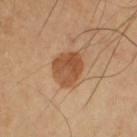biopsy status — imaged on a skin check; not biopsied
image-analysis metrics — a shape-asymmetry score of about 0.2 (0 = symmetric); an average lesion color of about L≈50 a*≈22 b*≈35 (CIELAB), about 11 CIELAB-L* units darker than the surrounding skin, and a normalized lesion–skin contrast near 8.5; a color-variation rating of about 5/10; an automated nevus-likeness rating near 90 out of 100 and lesion-presence confidence of about 100/100
subject — male, aged approximately 65
image — ~15 mm tile from a whole-body skin photo
tile lighting — cross-polarized illumination
site — the right upper arm